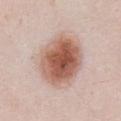- notes: no biopsy performed (imaged during a skin exam)
- body site: the front of the torso
- size: ≈7 mm
- automated lesion analysis: a border-irregularity index near 1.5/10, internal color variation of about 8 on a 0–10 scale, and peripheral color asymmetry of about 2; a nevus-likeness score of about 100/100 and a detector confidence of about 100 out of 100 that the crop contains a lesion
- subject: female, in their 40s
- image: 15 mm crop, total-body photography
- lighting: white-light